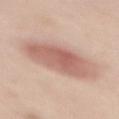Q: Was a biopsy performed?
A: catalogued during a skin exam; not biopsied
Q: Patient demographics?
A: female, roughly 50 years of age
Q: How was this image acquired?
A: 15 mm crop, total-body photography
Q: Illumination type?
A: white-light
Q: Where on the body is the lesion?
A: the mid back
Q: What did automated image analysis measure?
A: an eccentricity of roughly 0.8 and a shape-asymmetry score of about 0.15 (0 = symmetric); border irregularity of about 2 on a 0–10 scale; an automated nevus-likeness rating near 100 out of 100 and a detector confidence of about 100 out of 100 that the crop contains a lesion